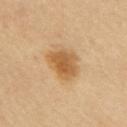Clinical impression: The lesion was tiled from a total-body skin photograph and was not biopsied. Context: The patient is a female approximately 40 years of age. This image is a 15 mm lesion crop taken from a total-body photograph. Approximately 4 mm at its widest. On the arm. An algorithmic analysis of the crop reported an eccentricity of roughly 0.6 and a shape-asymmetry score of about 0.25 (0 = symmetric). The analysis additionally found a mean CIELAB color near L≈59 a*≈19 b*≈41 and a lesion-to-skin contrast of about 8 (normalized; higher = more distinct). The software also gave border irregularity of about 2.5 on a 0–10 scale and radial color variation of about 1. The analysis additionally found an automated nevus-likeness rating near 90 out of 100. Captured under cross-polarized illumination.